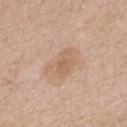Imaged during a routine full-body skin examination; the lesion was not biopsied and no histopathology is available.
From the chest.
A male subject aged 53 to 57.
Imaged with white-light lighting.
An algorithmic analysis of the crop reported an area of roughly 9.5 mm², an outline eccentricity of about 0.8 (0 = round, 1 = elongated), and two-axis asymmetry of about 0.3. It also reported a mean CIELAB color near L≈62 a*≈17 b*≈32, roughly 7 lightness units darker than nearby skin, and a lesion-to-skin contrast of about 5.5 (normalized; higher = more distinct).
A close-up tile cropped from a whole-body skin photograph, about 15 mm across.